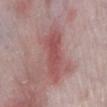{
  "lesion_size": {
    "long_diameter_mm_approx": 7.0
  },
  "image": {
    "source": "total-body photography crop",
    "field_of_view_mm": 15
  },
  "lighting": "white-light",
  "site": "left lower leg",
  "automated_metrics": {
    "area_mm2_approx": 13.0,
    "eccentricity": 0.95,
    "shape_asymmetry": 0.3,
    "border_irregularity_0_10": 4.0,
    "peripheral_color_asymmetry": 1.0,
    "nevus_likeness_0_100": 50,
    "lesion_detection_confidence_0_100": 95
  },
  "patient": {
    "sex": "male",
    "age_approx": 65
  }
}